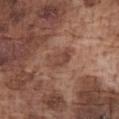This lesion was catalogued during total-body skin photography and was not selected for biopsy. The recorded lesion diameter is about 3.5 mm. The total-body-photography lesion software estimated a lesion area of about 5.5 mm², an outline eccentricity of about 0.85 (0 = round, 1 = elongated), and a symmetry-axis asymmetry near 0.3. And it measured about 7 CIELAB-L* units darker than the surrounding skin and a lesion-to-skin contrast of about 5.5 (normalized; higher = more distinct). From the front of the torso. Captured under white-light illumination. A region of skin cropped from a whole-body photographic capture, roughly 15 mm wide. A male subject, aged around 75.